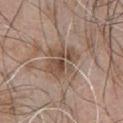Assessment: The lesion was tiled from a total-body skin photograph and was not biopsied. Image and clinical context: The patient is a male aged 48–52. The lesion is on the front of the torso. A 15 mm crop from a total-body photograph taken for skin-cancer surveillance. Longest diameter approximately 4 mm.